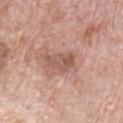Q: Is there a histopathology result?
A: imaged on a skin check; not biopsied
Q: Automated lesion metrics?
A: an area of roughly 6 mm², a shape eccentricity near 0.75, and two-axis asymmetry of about 0.3; border irregularity of about 4 on a 0–10 scale, internal color variation of about 4 on a 0–10 scale, and peripheral color asymmetry of about 1.5
Q: How was this image acquired?
A: ~15 mm tile from a whole-body skin photo
Q: Illumination type?
A: white-light
Q: What is the lesion's diameter?
A: about 3.5 mm
Q: What are the patient's age and sex?
A: male, in their mid-60s
Q: Where on the body is the lesion?
A: the chest